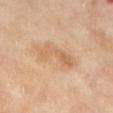Impression: This lesion was catalogued during total-body skin photography and was not selected for biopsy. Image and clinical context: The tile uses cross-polarized illumination. The subject is a female aged around 70. A 15 mm crop from a total-body photograph taken for skin-cancer surveillance. The lesion is on the left lower leg. Automated image analysis of the tile measured a shape-asymmetry score of about 0.45 (0 = symmetric). The analysis additionally found a lesion color around L≈65 a*≈20 b*≈36 in CIELAB, roughly 8 lightness units darker than nearby skin, and a lesion-to-skin contrast of about 5.5 (normalized; higher = more distinct). Measured at roughly 5 mm in maximum diameter.